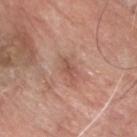Impression: The lesion was tiled from a total-body skin photograph and was not biopsied. Context: The lesion is on the head or neck. The patient is a male roughly 80 years of age. Cropped from a whole-body photographic skin survey; the tile spans about 15 mm.